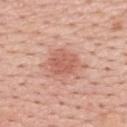Clinical impression: Part of a total-body skin-imaging series; this lesion was reviewed on a skin check and was not flagged for biopsy. Clinical summary: The tile uses white-light illumination. Longest diameter approximately 3.5 mm. A lesion tile, about 15 mm wide, cut from a 3D total-body photograph. A male patient, approximately 55 years of age. On the upper back.